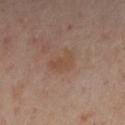Cropped from a total-body skin-imaging series; the visible field is about 15 mm. A female patient, roughly 40 years of age. The lesion is located on the left arm. This is a cross-polarized tile.